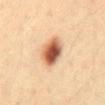{"biopsy_status": "not biopsied; imaged during a skin examination", "site": "abdomen", "image": {"source": "total-body photography crop", "field_of_view_mm": 15}, "patient": {"sex": "male", "age_approx": 40}, "automated_metrics": {"cielab_L": 53, "cielab_a": 23, "cielab_b": 32, "vs_skin_darker_L": 19.0, "vs_skin_contrast_norm": 12.0, "peripheral_color_asymmetry": 2.5, "nevus_likeness_0_100": 100}, "lighting": "cross-polarized"}